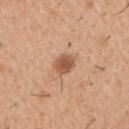Recorded during total-body skin imaging; not selected for excision or biopsy. Cropped from a total-body skin-imaging series; the visible field is about 15 mm. Imaged with white-light lighting. The lesion is on the right upper arm. About 2.5 mm across. The patient is a male roughly 40 years of age.